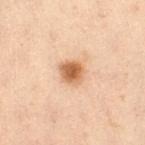Imaged during a routine full-body skin examination; the lesion was not biopsied and no histopathology is available. Located on the abdomen. A female patient about 55 years old. The total-body-photography lesion software estimated an average lesion color of about L≈52 a*≈19 b*≈32 (CIELAB), about 13 CIELAB-L* units darker than the surrounding skin, and a lesion-to-skin contrast of about 9.5 (normalized; higher = more distinct). The analysis additionally found a classifier nevus-likeness of about 100/100 and lesion-presence confidence of about 100/100. A 15 mm crop from a total-body photograph taken for skin-cancer surveillance. The lesion's longest dimension is about 3 mm. This is a cross-polarized tile.